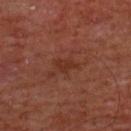workup: total-body-photography surveillance lesion; no biopsy | site: the chest | subject: male, in their 60s | imaging modality: total-body-photography crop, ~15 mm field of view.